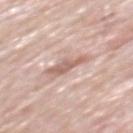Findings:
– location · the mid back
– image source · ~15 mm tile from a whole-body skin photo
– size · ~4 mm (longest diameter)
– patient · male, aged 78–82
– lighting · white-light illumination